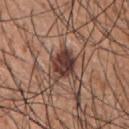biopsy status: total-body-photography surveillance lesion; no biopsy
patient: male, about 20 years old
size: ~4 mm (longest diameter)
imaging modality: ~15 mm tile from a whole-body skin photo
tile lighting: white-light illumination
automated lesion analysis: an area of roughly 9 mm², a shape eccentricity near 0.5, and a shape-asymmetry score of about 0.3 (0 = symmetric); a mean CIELAB color near L≈39 a*≈20 b*≈23 and a lesion–skin lightness drop of about 14; a border-irregularity rating of about 3.5/10 and radial color variation of about 1.5
body site: the upper back